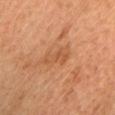This lesion was catalogued during total-body skin photography and was not selected for biopsy.
The tile uses cross-polarized illumination.
The total-body-photography lesion software estimated a normalized lesion–skin contrast near 5. And it measured a within-lesion color-variation index near 2.5/10 and radial color variation of about 0.5.
A female subject, aged 58–62.
A close-up tile cropped from a whole-body skin photograph, about 15 mm across.
Located on the head or neck.
The recorded lesion diameter is about 4 mm.